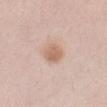Impression: The lesion was tiled from a total-body skin photograph and was not biopsied. Clinical summary: The lesion is located on the left forearm. A region of skin cropped from a whole-body photographic capture, roughly 15 mm wide. Automated tile analysis of the lesion measured an average lesion color of about L≈64 a*≈19 b*≈29 (CIELAB), about 10 CIELAB-L* units darker than the surrounding skin, and a normalized lesion–skin contrast near 7. And it measured border irregularity of about 1 on a 0–10 scale, internal color variation of about 2.5 on a 0–10 scale, and peripheral color asymmetry of about 1. The analysis additionally found a nevus-likeness score of about 95/100 and a detector confidence of about 100 out of 100 that the crop contains a lesion. Captured under white-light illumination. A female subject aged 38 to 42. The lesion's longest dimension is about 2.5 mm.